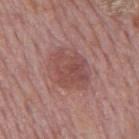Recorded during total-body skin imaging; not selected for excision or biopsy. The lesion is on the mid back. This image is a 15 mm lesion crop taken from a total-body photograph. The recorded lesion diameter is about 5.5 mm. A male patient about 75 years old. The tile uses white-light illumination.